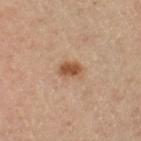Notes:
– anatomic site: the left thigh
– acquisition: ~15 mm tile from a whole-body skin photo
– illumination: cross-polarized illumination
– patient: male, aged around 65